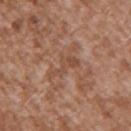Assessment:
The lesion was tiled from a total-body skin photograph and was not biopsied.
Acquisition and patient details:
The total-body-photography lesion software estimated a footprint of about 4 mm² and two-axis asymmetry of about 0.6. The software also gave an average lesion color of about L≈48 a*≈21 b*≈29 (CIELAB), roughly 7 lightness units darker than nearby skin, and a lesion-to-skin contrast of about 5.5 (normalized; higher = more distinct). It also reported a nevus-likeness score of about 0/100 and lesion-presence confidence of about 80/100. A male subject, roughly 45 years of age. Imaged with white-light lighting. On the right upper arm. A 15 mm crop from a total-body photograph taken for skin-cancer surveillance.